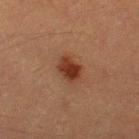{"biopsy_status": "not biopsied; imaged during a skin examination", "image": {"source": "total-body photography crop", "field_of_view_mm": 15}, "lesion_size": {"long_diameter_mm_approx": 3.0}, "patient": {"sex": "male", "age_approx": 40}, "automated_metrics": {"nevus_likeness_0_100": 100}, "site": "left thigh"}